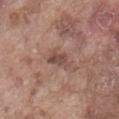Background: A male patient aged 73–77. The tile uses white-light illumination. An algorithmic analysis of the crop reported a lesion-to-skin contrast of about 7 (normalized; higher = more distinct). A 15 mm close-up extracted from a 3D total-body photography capture. Longest diameter approximately 3 mm. The lesion is on the abdomen.